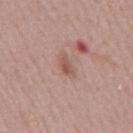biopsy_status: not biopsied; imaged during a skin examination
site: mid back
automated_metrics:
  area_mm2_approx: 3.0
  eccentricity: 0.9
  shape_asymmetry: 0.3
  cielab_L: 53
  cielab_a: 21
  cielab_b: 27
  vs_skin_darker_L: 9.0
  border_irregularity_0_10: 3.0
  color_variation_0_10: 0.5
  peripheral_color_asymmetry: 0.0
  nevus_likeness_0_100: 0
  lesion_detection_confidence_0_100: 100
image:
  source: total-body photography crop
  field_of_view_mm: 15
patient:
  sex: male
  age_approx: 55
lighting: white-light